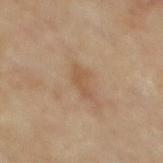• workup · no biopsy performed (imaged during a skin exam)
• acquisition · 15 mm crop, total-body photography
• site · the mid back
• subject · male, aged 83 or over
• lesion diameter · ~3 mm (longest diameter)
• tile lighting · cross-polarized